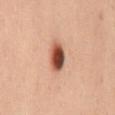Clinical impression:
The lesion was tiled from a total-body skin photograph and was not biopsied.
Clinical summary:
Measured at roughly 3.5 mm in maximum diameter. A male patient, about 40 years old. From the mid back. The tile uses cross-polarized illumination. The lesion-visualizer software estimated an outline eccentricity of about 0.7 (0 = round, 1 = elongated) and two-axis asymmetry of about 0.2. It also reported a nevus-likeness score of about 100/100. A 15 mm close-up extracted from a 3D total-body photography capture.